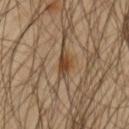image:
  source: total-body photography crop
  field_of_view_mm: 15
lighting: cross-polarized
patient:
  sex: male
  age_approx: 45
site: left upper arm
lesion_size:
  long_diameter_mm_approx: 4.0